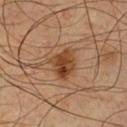A 15 mm crop from a total-body photograph taken for skin-cancer surveillance. The patient is a male aged approximately 40. This is a cross-polarized tile. The lesion is located on the chest. The recorded lesion diameter is about 4 mm.